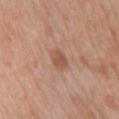<case>
  <biopsy_status>not biopsied; imaged during a skin examination</biopsy_status>
  <site>chest</site>
  <patient>
    <sex>female</sex>
    <age_approx>70</age_approx>
  </patient>
  <lesion_size>
    <long_diameter_mm_approx>2.5</long_diameter_mm_approx>
  </lesion_size>
  <image>
    <source>total-body photography crop</source>
    <field_of_view_mm>15</field_of_view_mm>
  </image>
  <lighting>white-light</lighting>
</case>